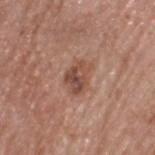The lesion was photographed on a routine skin check and not biopsied; there is no pathology result.
Located on the head or neck.
A region of skin cropped from a whole-body photographic capture, roughly 15 mm wide.
A male subject in their 60s.